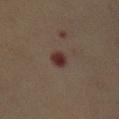workup: imaged on a skin check; not biopsied | lighting: cross-polarized illumination | image: total-body-photography crop, ~15 mm field of view | automated metrics: a border-irregularity index near 2/10, a within-lesion color-variation index near 3.5/10, and a peripheral color-asymmetry measure near 1.5 | patient: female, roughly 40 years of age | anatomic site: the abdomen | lesion size: about 3 mm.